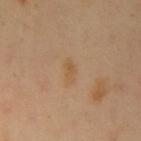- biopsy status · no biopsy performed (imaged during a skin exam)
- subject · male, aged 38–42
- anatomic site · the arm
- size · about 3 mm
- imaging modality · ~15 mm crop, total-body skin-cancer survey